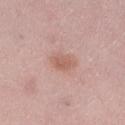Captured during whole-body skin photography for melanoma surveillance; the lesion was not biopsied.
The recorded lesion diameter is about 3 mm.
The tile uses white-light illumination.
The subject is a female in their mid-20s.
Cropped from a total-body skin-imaging series; the visible field is about 15 mm.
An algorithmic analysis of the crop reported a border-irregularity index near 2/10, internal color variation of about 2 on a 0–10 scale, and radial color variation of about 0.5. And it measured an automated nevus-likeness rating near 40 out of 100 and lesion-presence confidence of about 100/100.
Located on the right lower leg.